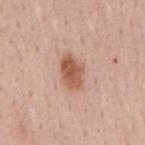No biopsy was performed on this lesion — it was imaged during a full skin examination and was not determined to be concerning.
The lesion is on the mid back.
A lesion tile, about 15 mm wide, cut from a 3D total-body photograph.
A male patient, in their mid- to late 50s.